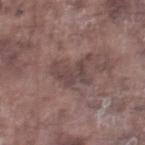biopsy_status: not biopsied; imaged during a skin examination
site: right thigh
image:
  source: total-body photography crop
  field_of_view_mm: 15
patient:
  sex: male
  age_approx: 75
lighting: white-light
lesion_size:
  long_diameter_mm_approx: 4.5